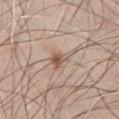{
  "biopsy_status": "not biopsied; imaged during a skin examination",
  "lighting": "white-light",
  "patient": {
    "sex": "male",
    "age_approx": 65
  },
  "site": "chest",
  "lesion_size": {
    "long_diameter_mm_approx": 3.0
  },
  "image": {
    "source": "total-body photography crop",
    "field_of_view_mm": 15
  }
}